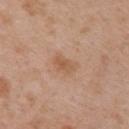biopsy_status: not biopsied; imaged during a skin examination
site: right upper arm
image:
  source: total-body photography crop
  field_of_view_mm: 15
patient:
  sex: female
  age_approx: 40
lesion_size:
  long_diameter_mm_approx: 2.5